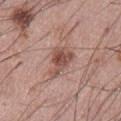Case summary:
- workup · total-body-photography surveillance lesion; no biopsy
- location · the abdomen
- acquisition · ~15 mm crop, total-body skin-cancer survey
- subject · male, in their mid-50s
- diameter · about 4 mm
- illumination · white-light
- image-analysis metrics · an area of roughly 8 mm² and two-axis asymmetry of about 0.4; a lesion color around L≈51 a*≈21 b*≈25 in CIELAB, about 11 CIELAB-L* units darker than the surrounding skin, and a normalized lesion–skin contrast near 8; a border-irregularity index near 4.5/10 and internal color variation of about 5 on a 0–10 scale